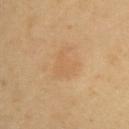Part of a total-body skin-imaging series; this lesion was reviewed on a skin check and was not flagged for biopsy. Captured under cross-polarized illumination. A female patient about 65 years old. Located on the left upper arm. The recorded lesion diameter is about 4 mm. A roughly 15 mm field-of-view crop from a total-body skin photograph.